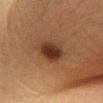<case>
<biopsy_status>not biopsied; imaged during a skin examination</biopsy_status>
<automated_metrics>
  <cielab_L>27</cielab_L>
  <cielab_a>19</cielab_a>
  <cielab_b>26</cielab_b>
  <vs_skin_darker_L>11.0</vs_skin_darker_L>
  <vs_skin_contrast_norm>10.5</vs_skin_contrast_norm>
</automated_metrics>
<lesion_size>
  <long_diameter_mm_approx>3.5</long_diameter_mm_approx>
</lesion_size>
<site>left lower leg</site>
<image>
  <source>total-body photography crop</source>
  <field_of_view_mm>15</field_of_view_mm>
</image>
<patient>
  <sex>female</sex>
  <age_approx>40</age_approx>
</patient>
<lighting>cross-polarized</lighting>
</case>